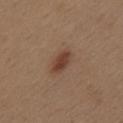Case summary:
* biopsy status: no biopsy performed (imaged during a skin exam)
* size: ≈3 mm
* subject: female, aged 38–42
* TBP lesion metrics: a lesion-detection confidence of about 100/100
* anatomic site: the back
* tile lighting: white-light
* image: ~15 mm tile from a whole-body skin photo